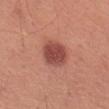Assessment: No biopsy was performed on this lesion — it was imaged during a full skin examination and was not determined to be concerning. Acquisition and patient details: The patient is a male about 65 years old. The tile uses white-light illumination. The lesion is on the left thigh. A 15 mm close-up tile from a total-body photography series done for melanoma screening. The total-body-photography lesion software estimated peripheral color asymmetry of about 1. And it measured a nevus-likeness score of about 95/100 and a lesion-detection confidence of about 100/100.